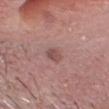Context:
Automated tile analysis of the lesion measured an eccentricity of roughly 0.8 and a shape-asymmetry score of about 0.2 (0 = symmetric). And it measured a border-irregularity rating of about 2/10 and a color-variation rating of about 1.5/10. The lesion is on the head or neck. Cropped from a whole-body photographic skin survey; the tile spans about 15 mm. A male patient aged 58 to 62. Captured under white-light illumination. Longest diameter approximately 2.5 mm.